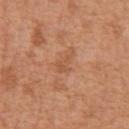  lighting: white-light
  patient:
    sex: male
    age_approx: 65
  image:
    source: total-body photography crop
    field_of_view_mm: 15
  site: abdomen